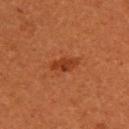<case>
  <biopsy_status>not biopsied; imaged during a skin examination</biopsy_status>
  <site>upper back</site>
  <lesion_size>
    <long_diameter_mm_approx>3.5</long_diameter_mm_approx>
  </lesion_size>
  <patient>
    <sex>female</sex>
    <age_approx>30</age_approx>
  </patient>
  <image>
    <source>total-body photography crop</source>
    <field_of_view_mm>15</field_of_view_mm>
  </image>
  <automated_metrics>
    <cielab_L>37</cielab_L>
    <cielab_a>29</cielab_a>
    <cielab_b>36</cielab_b>
    <vs_skin_contrast_norm>7.0</vs_skin_contrast_norm>
    <nevus_likeness_0_100>90</nevus_likeness_0_100>
    <lesion_detection_confidence_0_100>100</lesion_detection_confidence_0_100>
  </automated_metrics>
</case>